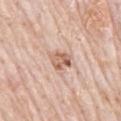follow-up: no biopsy performed (imaged during a skin exam)
lighting: white-light illumination
anatomic site: the mid back
image-analysis metrics: radial color variation of about 2
subject: male, approximately 80 years of age
image: ~15 mm tile from a whole-body skin photo
size: ~3 mm (longest diameter)